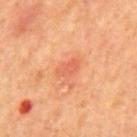<tbp_lesion>
  <biopsy_status>not biopsied; imaged during a skin examination</biopsy_status>
  <site>mid back</site>
  <patient>
    <sex>male</sex>
    <age_approx>65</age_approx>
  </patient>
  <lesion_size>
    <long_diameter_mm_approx>2.5</long_diameter_mm_approx>
  </lesion_size>
  <image>
    <source>total-body photography crop</source>
    <field_of_view_mm>15</field_of_view_mm>
  </image>
</tbp_lesion>